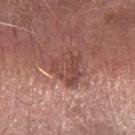Notes:
– follow-up: total-body-photography surveillance lesion; no biopsy
– imaging modality: ~15 mm crop, total-body skin-cancer survey
– site: the right forearm
– patient: male, about 65 years old